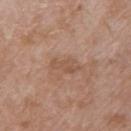Imaged during a routine full-body skin examination; the lesion was not biopsied and no histopathology is available.
A close-up tile cropped from a whole-body skin photograph, about 15 mm across.
A female patient aged approximately 70.
From the left upper arm.
Automated image analysis of the tile measured a footprint of about 4.5 mm², a shape eccentricity near 0.85, and two-axis asymmetry of about 0.4. The analysis additionally found roughly 7 lightness units darker than nearby skin and a normalized lesion–skin contrast near 5. The software also gave a within-lesion color-variation index near 2/10 and a peripheral color-asymmetry measure near 0.5.
The recorded lesion diameter is about 3.5 mm.